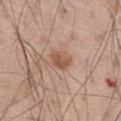Recorded during total-body skin imaging; not selected for excision or biopsy. On the right thigh. A male patient, aged 58–62. A 15 mm close-up tile from a total-body photography series done for melanoma screening. Longest diameter approximately 3 mm.